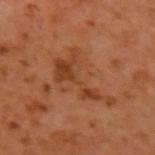anatomic site: the left upper arm
diameter: ~7 mm (longest diameter)
acquisition: ~15 mm crop, total-body skin-cancer survey
automated lesion analysis: a shape-asymmetry score of about 0.65 (0 = symmetric); a lesion color around L≈38 a*≈24 b*≈34 in CIELAB and about 8 CIELAB-L* units darker than the surrounding skin; a border-irregularity rating of about 10/10, a color-variation rating of about 3.5/10, and radial color variation of about 1
illumination: cross-polarized illumination
subject: male, in their 60s